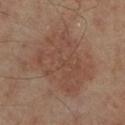follow-up=total-body-photography surveillance lesion; no biopsy
site=the left leg
subject=male, aged 48–52
diameter=about 9 mm
lighting=cross-polarized illumination
image=15 mm crop, total-body photography